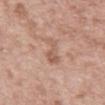imaging modality — 15 mm crop, total-body photography | diameter — ≈3.5 mm | illumination — white-light illumination | anatomic site — the mid back | TBP lesion metrics — an area of roughly 5 mm², an outline eccentricity of about 0.9 (0 = round, 1 = elongated), and two-axis asymmetry of about 0.4; an average lesion color of about L≈57 a*≈20 b*≈29 (CIELAB), about 8 CIELAB-L* units darker than the surrounding skin, and a lesion-to-skin contrast of about 5.5 (normalized; higher = more distinct); a border-irregularity index near 5/10 and radial color variation of about 0.5; a nevus-likeness score of about 0/100 and lesion-presence confidence of about 100/100 | patient — male, aged around 55.